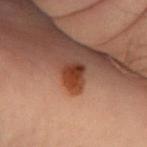biopsy_status: not biopsied; imaged during a skin examination
patient:
  sex: female
  age_approx: 50
image:
  source: total-body photography crop
  field_of_view_mm: 15
site: right forearm
automated_metrics:
  vs_skin_darker_L: 11.0
  vs_skin_contrast_norm: 10.0